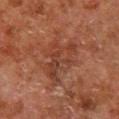Q: Was a biopsy performed?
A: total-body-photography surveillance lesion; no biopsy
Q: How was this image acquired?
A: 15 mm crop, total-body photography
Q: Illumination type?
A: cross-polarized illumination
Q: What are the patient's age and sex?
A: male, approximately 80 years of age
Q: Automated lesion metrics?
A: an average lesion color of about L≈31 a*≈20 b*≈24 (CIELAB) and a lesion–skin lightness drop of about 6
Q: Where on the body is the lesion?
A: the right lower leg